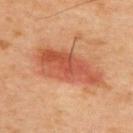| key | value |
|---|---|
| workup | imaged on a skin check; not biopsied |
| site | the upper back |
| subject | male, aged 43 to 47 |
| automated metrics | a lesion-to-skin contrast of about 8 (normalized; higher = more distinct); a classifier nevus-likeness of about 100/100 |
| lesion diameter | ~8.5 mm (longest diameter) |
| imaging modality | ~15 mm tile from a whole-body skin photo |
| lighting | cross-polarized illumination |